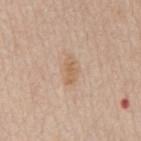{"biopsy_status": "not biopsied; imaged during a skin examination", "lighting": "white-light", "automated_metrics": {"area_mm2_approx": 4.5, "eccentricity": 0.85, "shape_asymmetry": 0.35, "border_irregularity_0_10": 3.5, "color_variation_0_10": 1.5, "peripheral_color_asymmetry": 0.5}, "image": {"source": "total-body photography crop", "field_of_view_mm": 15}, "lesion_size": {"long_diameter_mm_approx": 3.5}, "site": "mid back", "patient": {"sex": "male", "age_approx": 65}}